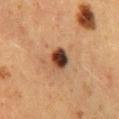Captured during whole-body skin photography for melanoma surveillance; the lesion was not biopsied. A female subject roughly 50 years of age. About 3 mm across. The lesion is on the back. The lesion-visualizer software estimated an area of roughly 6 mm², an eccentricity of roughly 0.65, and a symmetry-axis asymmetry near 0.2. A roughly 15 mm field-of-view crop from a total-body skin photograph.